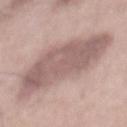Assessment: Imaged during a routine full-body skin examination; the lesion was not biopsied and no histopathology is available. Background: This is a white-light tile. Approximately 10.5 mm at its widest. A close-up tile cropped from a whole-body skin photograph, about 15 mm across. The lesion is on the back. Automated tile analysis of the lesion measured an eccentricity of roughly 0.85 and two-axis asymmetry of about 0.2. And it measured a mean CIELAB color near L≈58 a*≈17 b*≈20, about 12 CIELAB-L* units darker than the surrounding skin, and a lesion-to-skin contrast of about 7.5 (normalized; higher = more distinct). The software also gave a within-lesion color-variation index near 3/10 and a peripheral color-asymmetry measure near 1. It also reported a nevus-likeness score of about 15/100 and a detector confidence of about 95 out of 100 that the crop contains a lesion. A male patient, approximately 55 years of age.